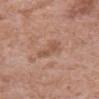Findings:
• notes · total-body-photography surveillance lesion; no biopsy
• image · ~15 mm tile from a whole-body skin photo
• anatomic site · the abdomen
• automated lesion analysis · a shape eccentricity near 0.85 and a shape-asymmetry score of about 0.4 (0 = symmetric); a mean CIELAB color near L≈53 a*≈22 b*≈29 and a lesion–skin lightness drop of about 8; a within-lesion color-variation index near 1.5/10 and peripheral color asymmetry of about 0.5; a nevus-likeness score of about 0/100
• subject · male, roughly 70 years of age